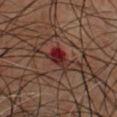Q: Was a biopsy performed?
A: imaged on a skin check; not biopsied
Q: Who is the patient?
A: male, in their mid-40s
Q: Automated lesion metrics?
A: an area of roughly 5 mm²; an automated nevus-likeness rating near 0 out of 100 and a detector confidence of about 100 out of 100 that the crop contains a lesion
Q: What kind of image is this?
A: ~15 mm crop, total-body skin-cancer survey
Q: What is the anatomic site?
A: the chest
Q: Illumination type?
A: cross-polarized
Q: What is the lesion's diameter?
A: ~3 mm (longest diameter)